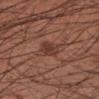workup=total-body-photography surveillance lesion; no biopsy